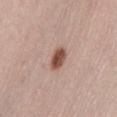workup = catalogued during a skin exam; not biopsied
body site = the abdomen
patient = female, roughly 50 years of age
diameter = about 3 mm
imaging modality = 15 mm crop, total-body photography